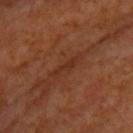Assessment:
Imaged during a routine full-body skin examination; the lesion was not biopsied and no histopathology is available.
Context:
A 15 mm close-up tile from a total-body photography series done for melanoma screening. A male patient in their mid-60s. From the upper back.